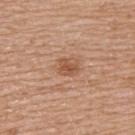Recorded during total-body skin imaging; not selected for excision or biopsy. A 15 mm crop from a total-body photograph taken for skin-cancer surveillance. Located on the upper back. Longest diameter approximately 2.5 mm. A female subject, approximately 70 years of age. The tile uses white-light illumination.